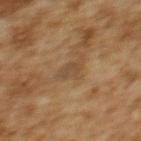Recorded during total-body skin imaging; not selected for excision or biopsy. On the upper back. Imaged with cross-polarized lighting. About 2.5 mm across. The subject is a female roughly 60 years of age. A 15 mm close-up extracted from a 3D total-body photography capture.